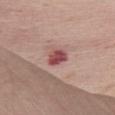This lesion was catalogued during total-body skin photography and was not selected for biopsy.
This is a white-light tile.
A region of skin cropped from a whole-body photographic capture, roughly 15 mm wide.
A female subject aged 63 to 67.
From the front of the torso.
The total-body-photography lesion software estimated a footprint of about 4.5 mm², a shape eccentricity near 0.55, and a symmetry-axis asymmetry near 0.2. The software also gave border irregularity of about 2 on a 0–10 scale, internal color variation of about 3.5 on a 0–10 scale, and peripheral color asymmetry of about 1.
About 2.5 mm across.